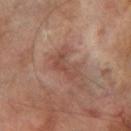{"biopsy_status": "not biopsied; imaged during a skin examination", "patient": {"sex": "male", "age_approx": 70}, "site": "left thigh", "lesion_size": {"long_diameter_mm_approx": 4.0}, "image": {"source": "total-body photography crop", "field_of_view_mm": 15}, "lighting": "cross-polarized"}